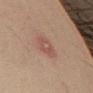{
  "lesion_size": {
    "long_diameter_mm_approx": 3.0
  },
  "image": {
    "source": "total-body photography crop",
    "field_of_view_mm": 15
  },
  "lighting": "cross-polarized",
  "site": "left upper arm",
  "patient": {
    "sex": "male",
    "age_approx": 50
  }
}